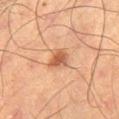<lesion>
  <biopsy_status>not biopsied; imaged during a skin examination</biopsy_status>
  <lighting>cross-polarized</lighting>
  <site>leg</site>
  <patient>
    <sex>male</sex>
    <age_approx>65</age_approx>
  </patient>
  <image>
    <source>total-body photography crop</source>
    <field_of_view_mm>15</field_of_view_mm>
  </image>
  <lesion_size>
    <long_diameter_mm_approx>2.5</long_diameter_mm_approx>
  </lesion_size>
</lesion>